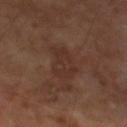Notes:
* patient — male, in their 70s
* anatomic site — the leg
* acquisition — 15 mm crop, total-body photography